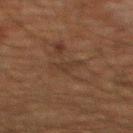Recorded during total-body skin imaging; not selected for excision or biopsy. The tile uses cross-polarized illumination. The total-body-photography lesion software estimated a border-irregularity rating of about 6.5/10 and a color-variation rating of about 0/10. It also reported a lesion-detection confidence of about 55/100. A 15 mm crop from a total-body photograph taken for skin-cancer surveillance. The lesion is located on the mid back. About 3 mm across. A male subject aged 58–62.